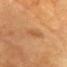This lesion was catalogued during total-body skin photography and was not selected for biopsy. A region of skin cropped from a whole-body photographic capture, roughly 15 mm wide. An algorithmic analysis of the crop reported a mean CIELAB color near L≈47 a*≈21 b*≈36 and a lesion-to-skin contrast of about 5 (normalized; higher = more distinct). The software also gave a border-irregularity index near 5.5/10, a within-lesion color-variation index near 0/10, and a peripheral color-asymmetry measure near 0. It also reported a classifier nevus-likeness of about 0/100 and a lesion-detection confidence of about 85/100. The recorded lesion diameter is about 3 mm. This is a cross-polarized tile. A male patient about 85 years old or older. From the head or neck.